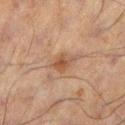Context:
An algorithmic analysis of the crop reported a footprint of about 4 mm², an outline eccentricity of about 0.7 (0 = round, 1 = elongated), and a symmetry-axis asymmetry near 0.3. The software also gave a lesion–skin lightness drop of about 8 and a normalized lesion–skin contrast near 7. The analysis additionally found a border-irregularity rating of about 3/10, internal color variation of about 3 on a 0–10 scale, and peripheral color asymmetry of about 1. And it measured a classifier nevus-likeness of about 25/100. The patient is a male aged 58 to 62. Measured at roughly 2.5 mm in maximum diameter. Captured under cross-polarized illumination. From the left lower leg. Cropped from a total-body skin-imaging series; the visible field is about 15 mm.